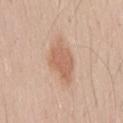Case summary:
• notes · no biopsy performed (imaged during a skin exam)
• patient · male, about 50 years old
• acquisition · ~15 mm tile from a whole-body skin photo
• body site · the lower back